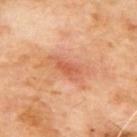This lesion was catalogued during total-body skin photography and was not selected for biopsy.
The tile uses cross-polarized illumination.
A 15 mm close-up tile from a total-body photography series done for melanoma screening.
The total-body-photography lesion software estimated an area of roughly 3.5 mm². And it measured a lesion–skin lightness drop of about 9 and a lesion-to-skin contrast of about 6 (normalized; higher = more distinct).
From the upper back.
The recorded lesion diameter is about 3 mm.
A male patient roughly 65 years of age.